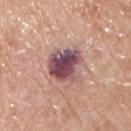This lesion was catalogued during total-body skin photography and was not selected for biopsy.
A lesion tile, about 15 mm wide, cut from a 3D total-body photograph.
The patient is a male aged around 65.
Imaged with white-light lighting.
From the chest.
Automated image analysis of the tile measured a lesion–skin lightness drop of about 18 and a normalized border contrast of about 13.5. And it measured a peripheral color-asymmetry measure near 2.